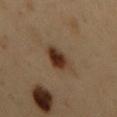workup: imaged on a skin check; not biopsied
tile lighting: cross-polarized
anatomic site: the mid back
image-analysis metrics: a footprint of about 7 mm², a shape eccentricity near 0.8, and a symmetry-axis asymmetry near 0.2; a lesion color around L≈33 a*≈17 b*≈28 in CIELAB, a lesion–skin lightness drop of about 13, and a normalized border contrast of about 12; a border-irregularity rating of about 2/10, a within-lesion color-variation index near 5.5/10, and a peripheral color-asymmetry measure near 1.5
acquisition: ~15 mm crop, total-body skin-cancer survey
patient: male, about 50 years old